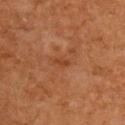Part of a total-body skin-imaging series; this lesion was reviewed on a skin check and was not flagged for biopsy. A close-up tile cropped from a whole-body skin photograph, about 15 mm across. Imaged with cross-polarized lighting. A male subject roughly 60 years of age. About 2 mm across. Located on the upper back.